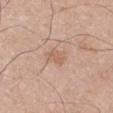Recorded during total-body skin imaging; not selected for excision or biopsy.
The subject is a male in their mid-60s.
The lesion is located on the left upper arm.
Cropped from a total-body skin-imaging series; the visible field is about 15 mm.
Approximately 3 mm at its widest.
Captured under white-light illumination.